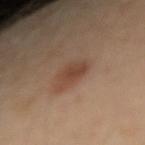Recorded during total-body skin imaging; not selected for excision or biopsy.
Captured under cross-polarized illumination.
The subject is a female roughly 40 years of age.
A close-up tile cropped from a whole-body skin photograph, about 15 mm across.
On the left arm.